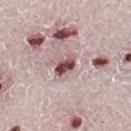<record>
  <biopsy_status>not biopsied; imaged during a skin examination</biopsy_status>
  <automated_metrics>
    <lesion_detection_confidence_0_100>100</lesion_detection_confidence_0_100>
  </automated_metrics>
  <patient>
    <sex>male</sex>
    <age_approx>65</age_approx>
  </patient>
  <site>right lower leg</site>
  <image>
    <source>total-body photography crop</source>
    <field_of_view_mm>15</field_of_view_mm>
  </image>
  <lesion_size>
    <long_diameter_mm_approx>3.0</long_diameter_mm_approx>
  </lesion_size>
  <lighting>white-light</lighting>
</record>